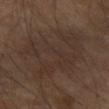Case summary:
- patient: male, roughly 65 years of age
- lesion diameter: about 11 mm
- anatomic site: the left arm
- image: 15 mm crop, total-body photography